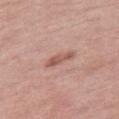Assessment: Captured during whole-body skin photography for melanoma surveillance; the lesion was not biopsied. Acquisition and patient details: The recorded lesion diameter is about 3.5 mm. The lesion is on the leg. The subject is a female approximately 70 years of age. A 15 mm close-up tile from a total-body photography series done for melanoma screening. The total-body-photography lesion software estimated a classifier nevus-likeness of about 5/100 and lesion-presence confidence of about 100/100. This is a white-light tile.